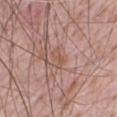notes: imaged on a skin check; not biopsied
imaging modality: ~15 mm crop, total-body skin-cancer survey
body site: the chest
tile lighting: white-light illumination
size: ≈2.5 mm
subject: male, aged 68 to 72
image-analysis metrics: a footprint of about 3 mm² and an outline eccentricity of about 0.9 (0 = round, 1 = elongated); a mean CIELAB color near L≈54 a*≈21 b*≈27 and a lesion-to-skin contrast of about 5.5 (normalized; higher = more distinct)